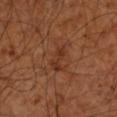Part of a total-body skin-imaging series; this lesion was reviewed on a skin check and was not flagged for biopsy. The patient is aged approximately 65. The lesion's longest dimension is about 3.5 mm. Automated image analysis of the tile measured an area of roughly 5 mm² and an eccentricity of roughly 0.9. And it measured a nevus-likeness score of about 0/100. The lesion is on the left forearm. Cropped from a total-body skin-imaging series; the visible field is about 15 mm. Imaged with cross-polarized lighting.